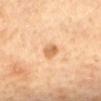Q: Was a biopsy performed?
A: no biopsy performed (imaged during a skin exam)
Q: Who is the patient?
A: female, aged approximately 65
Q: What is the anatomic site?
A: the mid back
Q: Illumination type?
A: cross-polarized illumination
Q: What did automated image analysis measure?
A: a mean CIELAB color near L≈64 a*≈21 b*≈40, about 11 CIELAB-L* units darker than the surrounding skin, and a lesion-to-skin contrast of about 7.5 (normalized; higher = more distinct); a border-irregularity index near 2/10, internal color variation of about 2.5 on a 0–10 scale, and radial color variation of about 1
Q: How was this image acquired?
A: total-body-photography crop, ~15 mm field of view